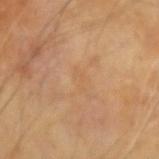Notes:
• follow-up — no biopsy performed (imaged during a skin exam)
• body site — the left forearm
• automated metrics — an area of roughly 2.5 mm², a shape eccentricity near 0.9, and a shape-asymmetry score of about 0.4 (0 = symmetric); a lesion color around L≈57 a*≈18 b*≈36 in CIELAB and a lesion-to-skin contrast of about 3 (normalized; higher = more distinct); a border-irregularity index near 4.5/10, a color-variation rating of about 0/10, and a peripheral color-asymmetry measure near 0
• image — total-body-photography crop, ~15 mm field of view
• lighting — cross-polarized
• lesion diameter — ≈2.5 mm
• subject — male, aged 68 to 72